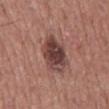Imaged during a routine full-body skin examination; the lesion was not biopsied and no histopathology is available. The lesion's longest dimension is about 5 mm. The lesion is on the mid back. This is a white-light tile. A male patient aged approximately 75. This image is a 15 mm lesion crop taken from a total-body photograph.